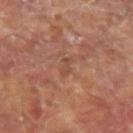Notes:
– location — the left forearm
– imaging modality — total-body-photography crop, ~15 mm field of view
– lighting — cross-polarized illumination
– patient — male, about 65 years old
– size — ≈2.5 mm